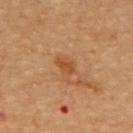This lesion was catalogued during total-body skin photography and was not selected for biopsy.
This image is a 15 mm lesion crop taken from a total-body photograph.
The patient is approximately 60 years of age.
Located on the upper back.